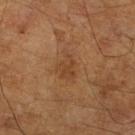- biopsy status: total-body-photography surveillance lesion; no biopsy
- lesion diameter: ≈4 mm
- patient: male, aged 63–67
- image-analysis metrics: an automated nevus-likeness rating near 15 out of 100
- imaging modality: ~15 mm tile from a whole-body skin photo